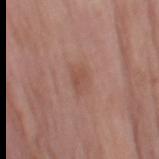Imaged during a routine full-body skin examination; the lesion was not biopsied and no histopathology is available.